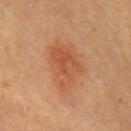Recorded during total-body skin imaging; not selected for excision or biopsy. From the chest. A 15 mm crop from a total-body photograph taken for skin-cancer surveillance. A female subject aged approximately 60. Measured at roughly 7 mm in maximum diameter.